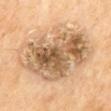location: the mid back | image: 15 mm crop, total-body photography | patient: male, approximately 70 years of age.